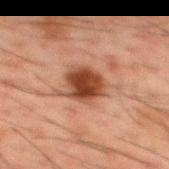No biopsy was performed on this lesion — it was imaged during a full skin examination and was not determined to be concerning. A 15 mm close-up tile from a total-body photography series done for melanoma screening. This is a cross-polarized tile. The lesion-visualizer software estimated an area of roughly 11 mm², an eccentricity of roughly 0.6, and a symmetry-axis asymmetry near 0.25. It also reported border irregularity of about 3 on a 0–10 scale, a color-variation rating of about 3.5/10, and radial color variation of about 1. A male subject, aged 48–52. On the back.